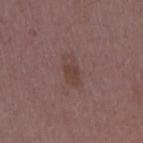Case summary:
– follow-up — imaged on a skin check; not biopsied
– subject — female, approximately 45 years of age
– acquisition — 15 mm crop, total-body photography
– site — the right upper arm
– lesion size — about 3.5 mm